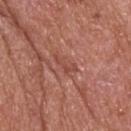{
  "biopsy_status": "not biopsied; imaged during a skin examination",
  "automated_metrics": {
    "area_mm2_approx": 3.0,
    "eccentricity": 0.95,
    "shape_asymmetry": 0.5,
    "cielab_L": 48,
    "cielab_a": 27,
    "cielab_b": 28,
    "vs_skin_darker_L": 7.0,
    "color_variation_0_10": 0.0,
    "peripheral_color_asymmetry": 0.0,
    "lesion_detection_confidence_0_100": 55
  },
  "site": "head or neck",
  "image": {
    "source": "total-body photography crop",
    "field_of_view_mm": 15
  },
  "lesion_size": {
    "long_diameter_mm_approx": 3.5
  },
  "patient": {
    "sex": "male",
    "age_approx": 65
  },
  "lighting": "white-light"
}